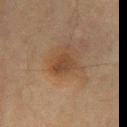Q: Is there a histopathology result?
A: catalogued during a skin exam; not biopsied
Q: Lesion size?
A: ~3 mm (longest diameter)
Q: How was this image acquired?
A: 15 mm crop, total-body photography
Q: Illumination type?
A: cross-polarized
Q: Patient demographics?
A: male, about 65 years old
Q: What is the anatomic site?
A: the chest
Q: What did automated image analysis measure?
A: a mean CIELAB color near L≈33 a*≈16 b*≈26, about 7 CIELAB-L* units darker than the surrounding skin, and a lesion-to-skin contrast of about 7 (normalized; higher = more distinct); border irregularity of about 2.5 on a 0–10 scale, a color-variation rating of about 2.5/10, and a peripheral color-asymmetry measure near 1